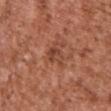Captured during whole-body skin photography for melanoma surveillance; the lesion was not biopsied. Captured under white-light illumination. The lesion is on the upper back. Cropped from a total-body skin-imaging series; the visible field is about 15 mm. An algorithmic analysis of the crop reported a mean CIELAB color near L≈44 a*≈26 b*≈30, a lesion–skin lightness drop of about 8, and a normalized border contrast of about 6.5. The analysis additionally found a border-irregularity index near 3/10, a within-lesion color-variation index near 3.5/10, and radial color variation of about 1. The patient is a male aged around 75. Approximately 3 mm at its widest.